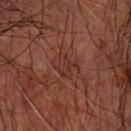body site — the right forearm
image — 15 mm crop, total-body photography
lighting — cross-polarized
diameter — ≈2.5 mm
patient — male, about 70 years old
image-analysis metrics — an average lesion color of about L≈26 a*≈18 b*≈22 (CIELAB) and about 4 CIELAB-L* units darker than the surrounding skin; a border-irregularity rating of about 5.5/10 and internal color variation of about 1 on a 0–10 scale